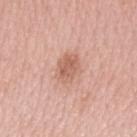Recorded during total-body skin imaging; not selected for excision or biopsy. A roughly 15 mm field-of-view crop from a total-body skin photograph. Located on the left upper arm. Longest diameter approximately 3.5 mm. The patient is a female aged 68 to 72.